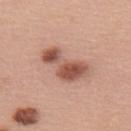This lesion was catalogued during total-body skin photography and was not selected for biopsy.
The lesion is on the upper back.
The total-body-photography lesion software estimated an outline eccentricity of about 0.85 (0 = round, 1 = elongated) and two-axis asymmetry of about 0.5. The software also gave a border-irregularity index near 5.5/10, a color-variation rating of about 7/10, and a peripheral color-asymmetry measure near 2.5.
A region of skin cropped from a whole-body photographic capture, roughly 15 mm wide.
This is a white-light tile.
A female subject about 60 years old.
Approximately 5.5 mm at its widest.